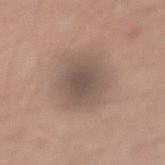Context: The lesion is located on the right forearm. The patient is a male aged approximately 40. The recorded lesion diameter is about 5 mm. An algorithmic analysis of the crop reported a lesion color around L≈51 a*≈13 b*≈22 in CIELAB, about 9 CIELAB-L* units darker than the surrounding skin, and a normalized lesion–skin contrast near 7. It also reported border irregularity of about 1.5 on a 0–10 scale. It also reported a nevus-likeness score of about 5/100 and a detector confidence of about 85 out of 100 that the crop contains a lesion. Cropped from a whole-body photographic skin survey; the tile spans about 15 mm.